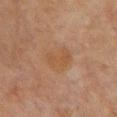workup=no biopsy performed (imaged during a skin exam)
lighting=cross-polarized
image source=total-body-photography crop, ~15 mm field of view
body site=the chest
automated metrics=a footprint of about 9 mm²; a nevus-likeness score of about 0/100 and a detector confidence of about 100 out of 100 that the crop contains a lesion
subject=female, aged approximately 70
lesion size=about 3.5 mm